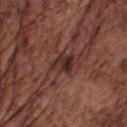  biopsy_status: not biopsied; imaged during a skin examination
  patient:
    sex: male
    age_approx: 75
  lesion_size:
    long_diameter_mm_approx: 3.0
  lighting: white-light
  image:
    source: total-body photography crop
    field_of_view_mm: 15
  site: front of the torso